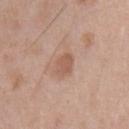Clinical impression: Part of a total-body skin-imaging series; this lesion was reviewed on a skin check and was not flagged for biopsy. Background: A male patient, about 65 years old. An algorithmic analysis of the crop reported a lesion–skin lightness drop of about 9 and a normalized border contrast of about 6.5. The lesion is on the chest. A 15 mm crop from a total-body photograph taken for skin-cancer surveillance. This is a white-light tile. Measured at roughly 3 mm in maximum diameter.